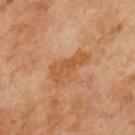biopsy_status: not biopsied; imaged during a skin examination
image:
  source: total-body photography crop
  field_of_view_mm: 15
lesion_size:
  long_diameter_mm_approx: 5.0
patient:
  sex: male
  age_approx: 65
lighting: cross-polarized